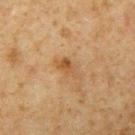Background:
The lesion is located on the left upper arm. Measured at roughly 3 mm in maximum diameter. An algorithmic analysis of the crop reported a lesion–skin lightness drop of about 7. And it measured a border-irregularity index near 4/10 and a peripheral color-asymmetry measure near 1. A roughly 15 mm field-of-view crop from a total-body skin photograph. A male patient, aged 58 to 62.